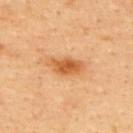Notes:
* biopsy status — catalogued during a skin exam; not biopsied
* body site — the back
* imaging modality — total-body-photography crop, ~15 mm field of view
* subject — male, aged 63–67